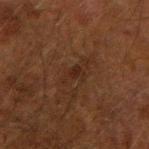Clinical summary: Automated image analysis of the tile measured an average lesion color of about L≈19 a*≈15 b*≈21 (CIELAB), roughly 4 lightness units darker than nearby skin, and a normalized border contrast of about 6. The analysis additionally found border irregularity of about 4.5 on a 0–10 scale, a color-variation rating of about 1/10, and a peripheral color-asymmetry measure near 0.5. It also reported a nevus-likeness score of about 0/100. From the right upper arm. The subject is a male approximately 50 years of age. Cropped from a total-body skin-imaging series; the visible field is about 15 mm.